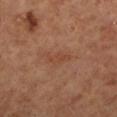follow-up: catalogued during a skin exam; not biopsied
illumination: cross-polarized illumination
anatomic site: the leg
automated lesion analysis: a footprint of about 3.5 mm², an outline eccentricity of about 0.85 (0 = round, 1 = elongated), and two-axis asymmetry of about 0.3; a lesion color around L≈43 a*≈22 b*≈31 in CIELAB and a normalized lesion–skin contrast near 5
patient: female, about 65 years old
image: ~15 mm crop, total-body skin-cancer survey